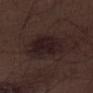Impression: Recorded during total-body skin imaging; not selected for excision or biopsy.